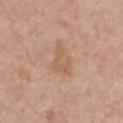Image and clinical context:
The lesion is on the chest. The patient is a female aged around 40. A roughly 15 mm field-of-view crop from a total-body skin photograph.